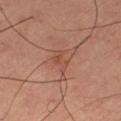Assessment:
The lesion was tiled from a total-body skin photograph and was not biopsied.
Image and clinical context:
Captured under cross-polarized illumination. A male subject, aged 63 to 67. From the left thigh. The recorded lesion diameter is about 3 mm. A lesion tile, about 15 mm wide, cut from a 3D total-body photograph. An algorithmic analysis of the crop reported an area of roughly 4 mm², a shape eccentricity near 0.85, and a shape-asymmetry score of about 0.35 (0 = symmetric). The analysis additionally found a border-irregularity rating of about 4.5/10 and a within-lesion color-variation index near 2.5/10. It also reported a lesion-detection confidence of about 100/100.